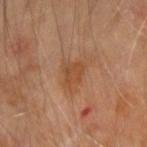Impression:
This lesion was catalogued during total-body skin photography and was not selected for biopsy.
Clinical summary:
From the right forearm. Longest diameter approximately 3 mm. A roughly 15 mm field-of-view crop from a total-body skin photograph. A male patient aged 68–72. The tile uses cross-polarized illumination.